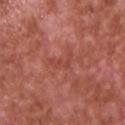– biopsy status · no biopsy performed (imaged during a skin exam)
– lesion size · about 3 mm
– anatomic site · the chest
– subject · male, in their mid- to late 60s
– acquisition · ~15 mm tile from a whole-body skin photo
– lighting · white-light illumination
– TBP lesion metrics · an eccentricity of roughly 0.9; a mean CIELAB color near L≈46 a*≈31 b*≈29, about 6 CIELAB-L* units darker than the surrounding skin, and a normalized lesion–skin contrast near 4.5; border irregularity of about 8 on a 0–10 scale and internal color variation of about 0 on a 0–10 scale; a lesion-detection confidence of about 90/100